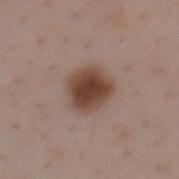biopsy_status: not biopsied; imaged during a skin examination
site: mid back
lesion_size:
  long_diameter_mm_approx: 4.0
lighting: white-light
image:
  source: total-body photography crop
  field_of_view_mm: 15
automated_metrics:
  area_mm2_approx: 13.0
  shape_asymmetry: 0.15
  cielab_L: 44
  cielab_a: 18
  cielab_b: 25
  vs_skin_contrast_norm: 10.5
patient:
  sex: female
  age_approx: 45